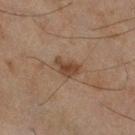follow-up: imaged on a skin check; not biopsied
tile lighting: cross-polarized
acquisition: total-body-photography crop, ~15 mm field of view
anatomic site: the right lower leg
lesion size: about 3 mm
patient: male, aged approximately 45